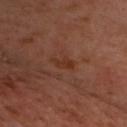| feature | finding |
|---|---|
| workup | catalogued during a skin exam; not biopsied |
| location | the front of the torso |
| image | ~15 mm tile from a whole-body skin photo |
| subject | male, aged 53 to 57 |